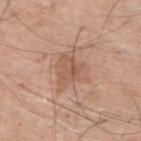Recorded during total-body skin imaging; not selected for excision or biopsy. This is a white-light tile. A male subject, aged 68 to 72. The lesion is located on the back. The lesion-visualizer software estimated a shape-asymmetry score of about 0.4 (0 = symmetric). A 15 mm close-up tile from a total-body photography series done for melanoma screening. Measured at roughly 5 mm in maximum diameter.